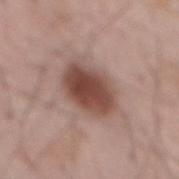follow-up: catalogued during a skin exam; not biopsied
tile lighting: white-light
image: total-body-photography crop, ~15 mm field of view
lesion size: about 6 mm
patient: male, roughly 65 years of age
automated lesion analysis: a lesion area of about 17 mm², an outline eccentricity of about 0.8 (0 = round, 1 = elongated), and a shape-asymmetry score of about 0.15 (0 = symmetric); border irregularity of about 1.5 on a 0–10 scale, a within-lesion color-variation index near 5/10, and radial color variation of about 1.5; a classifier nevus-likeness of about 100/100
site: the mid back